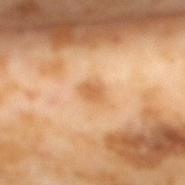The lesion was photographed on a routine skin check and not biopsied; there is no pathology result. A female subject, in their mid- to late 50s. The total-body-photography lesion software estimated a lesion color around L≈65 a*≈23 b*≈43 in CIELAB. It also reported a border-irregularity index near 2/10, a color-variation rating of about 1.5/10, and a peripheral color-asymmetry measure near 0.5. The analysis additionally found a nevus-likeness score of about 0/100 and lesion-presence confidence of about 100/100. A close-up tile cropped from a whole-body skin photograph, about 15 mm across. The lesion is on the mid back. The tile uses cross-polarized illumination.